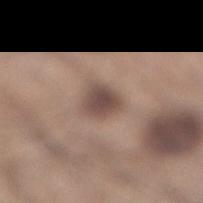follow-up — imaged on a skin check; not biopsied | diameter — ≈3 mm | image source — total-body-photography crop, ~15 mm field of view | subject — male, in their mid- to late 30s | body site — the right lower leg | illumination — white-light | TBP lesion metrics — an area of roughly 6.5 mm², an outline eccentricity of about 0.4 (0 = round, 1 = elongated), and a shape-asymmetry score of about 0.3 (0 = symmetric); border irregularity of about 2.5 on a 0–10 scale, a within-lesion color-variation index near 3/10, and radial color variation of about 0.5; a classifier nevus-likeness of about 30/100 and lesion-presence confidence of about 100/100.